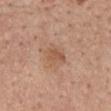follow-up = total-body-photography surveillance lesion; no biopsy | patient = male, aged 53 to 57 | diameter = ~2.5 mm (longest diameter) | automated lesion analysis = a lesion color around L≈54 a*≈21 b*≈32 in CIELAB and a lesion–skin lightness drop of about 8 | location = the mid back | illumination = white-light illumination | image = ~15 mm tile from a whole-body skin photo.